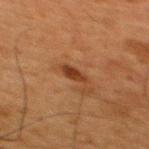| feature | finding |
|---|---|
| follow-up | no biopsy performed (imaged during a skin exam) |
| image | ~15 mm tile from a whole-body skin photo |
| patient | male, roughly 65 years of age |
| lesion diameter | ≈3 mm |
| lighting | cross-polarized illumination |
| location | the upper back |